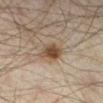Assessment:
No biopsy was performed on this lesion — it was imaged during a full skin examination and was not determined to be concerning.
Acquisition and patient details:
Cropped from a whole-body photographic skin survey; the tile spans about 15 mm. Measured at roughly 4 mm in maximum diameter. The lesion is on the right lower leg. Automated image analysis of the tile measured an area of roughly 7.5 mm², a shape eccentricity near 0.8, and a shape-asymmetry score of about 0.25 (0 = symmetric). It also reported an average lesion color of about L≈41 a*≈13 b*≈25 (CIELAB), roughly 10 lightness units darker than nearby skin, and a normalized border contrast of about 9. Imaged with cross-polarized lighting. A male patient, approximately 45 years of age.